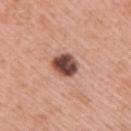Case summary:
* biopsy status · imaged on a skin check; not biopsied
* automated lesion analysis · an area of roughly 7.5 mm², an outline eccentricity of about 0.6 (0 = round, 1 = elongated), and a symmetry-axis asymmetry near 0.15
* patient · male, aged around 60
* site · the right upper arm
* illumination · white-light
* acquisition · total-body-photography crop, ~15 mm field of view